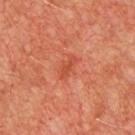• workup · no biopsy performed (imaged during a skin exam)
• size · ~3 mm (longest diameter)
• subject · male, aged approximately 60
• image-analysis metrics · an area of roughly 4 mm², a shape eccentricity near 0.9, and a shape-asymmetry score of about 0.3 (0 = symmetric); a lesion color around L≈48 a*≈33 b*≈36 in CIELAB, roughly 7 lightness units darker than nearby skin, and a lesion-to-skin contrast of about 5.5 (normalized; higher = more distinct); lesion-presence confidence of about 100/100
• image source · total-body-photography crop, ~15 mm field of view
• anatomic site · the chest